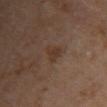The lesion was tiled from a total-body skin photograph and was not biopsied.
The lesion's longest dimension is about 2.5 mm.
This is a cross-polarized tile.
A 15 mm close-up tile from a total-body photography series done for melanoma screening.
A female subject, approximately 60 years of age.
The lesion is located on the chest.
An algorithmic analysis of the crop reported an area of roughly 3.5 mm², an eccentricity of roughly 0.75, and a symmetry-axis asymmetry near 0.35. The software also gave an average lesion color of about L≈34 a*≈16 b*≈26 (CIELAB).